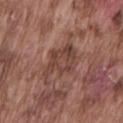Q: Was a biopsy performed?
A: imaged on a skin check; not biopsied
Q: What did automated image analysis measure?
A: a lesion area of about 12 mm² and two-axis asymmetry of about 0.35; a mean CIELAB color near L≈42 a*≈21 b*≈24 and a lesion–skin lightness drop of about 8; a border-irregularity index near 4.5/10 and a color-variation rating of about 4/10; an automated nevus-likeness rating near 0 out of 100 and a detector confidence of about 60 out of 100 that the crop contains a lesion
Q: What is the lesion's diameter?
A: about 5 mm
Q: Where on the body is the lesion?
A: the lower back
Q: How was the tile lit?
A: white-light
Q: What are the patient's age and sex?
A: male, approximately 75 years of age
Q: How was this image acquired?
A: total-body-photography crop, ~15 mm field of view